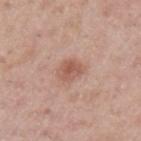Assessment:
Recorded during total-body skin imaging; not selected for excision or biopsy.
Acquisition and patient details:
A region of skin cropped from a whole-body photographic capture, roughly 15 mm wide. Automated image analysis of the tile measured a footprint of about 5.5 mm², an outline eccentricity of about 0.25 (0 = round, 1 = elongated), and a shape-asymmetry score of about 0.25 (0 = symmetric). The software also gave an average lesion color of about L≈56 a*≈22 b*≈28 (CIELAB), about 10 CIELAB-L* units darker than the surrounding skin, and a normalized border contrast of about 7. It also reported border irregularity of about 2.5 on a 0–10 scale, a color-variation rating of about 3/10, and peripheral color asymmetry of about 1. The recorded lesion diameter is about 2.5 mm. This is a white-light tile. A male patient roughly 40 years of age. Located on the left upper arm.